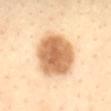Q: Is there a histopathology result?
A: catalogued during a skin exam; not biopsied
Q: How was this image acquired?
A: ~15 mm tile from a whole-body skin photo
Q: Lesion location?
A: the mid back
Q: What are the patient's age and sex?
A: female, roughly 40 years of age
Q: How was the tile lit?
A: cross-polarized illumination